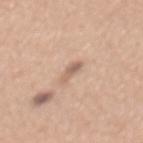Assessment:
The lesion was tiled from a total-body skin photograph and was not biopsied.
Context:
A female subject approximately 30 years of age. Longest diameter approximately 3 mm. This is a white-light tile. The lesion is on the mid back. The total-body-photography lesion software estimated a mean CIELAB color near L≈61 a*≈18 b*≈28 and a lesion-to-skin contrast of about 6.5 (normalized; higher = more distinct). It also reported a border-irregularity rating of about 4/10, a color-variation rating of about 0/10, and radial color variation of about 0. A close-up tile cropped from a whole-body skin photograph, about 15 mm across.